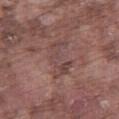Recorded during total-body skin imaging; not selected for excision or biopsy.
This is a white-light tile.
Measured at roughly 4.5 mm in maximum diameter.
Cropped from a total-body skin-imaging series; the visible field is about 15 mm.
A male patient in their mid-70s.
The lesion is located on the right thigh.